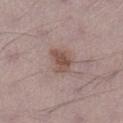Q: Was this lesion biopsied?
A: catalogued during a skin exam; not biopsied
Q: What kind of image is this?
A: 15 mm crop, total-body photography
Q: Lesion location?
A: the left lower leg
Q: What lighting was used for the tile?
A: white-light
Q: What is the lesion's diameter?
A: about 3 mm
Q: Who is the patient?
A: male, roughly 70 years of age